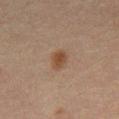Imaged during a routine full-body skin examination; the lesion was not biopsied and no histopathology is available. Automated tile analysis of the lesion measured a footprint of about 4.5 mm², a shape eccentricity near 0.75, and a shape-asymmetry score of about 0.25 (0 = symmetric). The software also gave a border-irregularity index near 2/10. A 15 mm close-up tile from a total-body photography series done for melanoma screening. On the front of the torso. A male subject aged around 50. Captured under cross-polarized illumination.